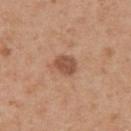follow-up = total-body-photography surveillance lesion; no biopsy | body site = the left upper arm | subject = female, aged approximately 40 | illumination = white-light | image source = ~15 mm crop, total-body skin-cancer survey.